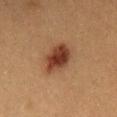Impression:
Imaged during a routine full-body skin examination; the lesion was not biopsied and no histopathology is available.
Context:
The lesion's longest dimension is about 5 mm. A close-up tile cropped from a whole-body skin photograph, about 15 mm across. The lesion-visualizer software estimated a footprint of about 10 mm², a shape eccentricity near 0.8, and a symmetry-axis asymmetry near 0.2. The software also gave a classifier nevus-likeness of about 100/100 and lesion-presence confidence of about 100/100. Imaged with cross-polarized lighting. The lesion is located on the chest. A male subject, roughly 50 years of age.